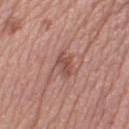notes = no biopsy performed (imaged during a skin exam)
image source = ~15 mm tile from a whole-body skin photo
lesion diameter = ≈3 mm
body site = the left thigh
subject = female, aged approximately 65
lighting = white-light